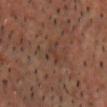{
  "biopsy_status": "not biopsied; imaged during a skin examination",
  "automated_metrics": {
    "eccentricity": 0.75,
    "peripheral_color_asymmetry": 0.0
  },
  "patient": {
    "sex": "male",
    "age_approx": 55
  },
  "image": {
    "source": "total-body photography crop",
    "field_of_view_mm": 15
  },
  "site": "head or neck",
  "lighting": "cross-polarized",
  "lesion_size": {
    "long_diameter_mm_approx": 3.0
  }
}